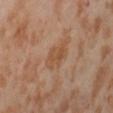This lesion was catalogued during total-body skin photography and was not selected for biopsy. A female subject, about 55 years old. A 15 mm close-up extracted from a 3D total-body photography capture. From the abdomen. Captured under cross-polarized illumination.